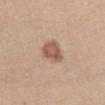No biopsy was performed on this lesion — it was imaged during a full skin examination and was not determined to be concerning.